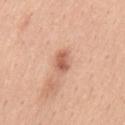{"biopsy_status": "not biopsied; imaged during a skin examination", "patient": {"sex": "male", "age_approx": 40}, "site": "mid back", "image": {"source": "total-body photography crop", "field_of_view_mm": 15}, "automated_metrics": {"shape_asymmetry": 0.2, "border_irregularity_0_10": 2.0, "peripheral_color_asymmetry": 1.0, "nevus_likeness_0_100": 55, "lesion_detection_confidence_0_100": 100}}